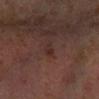Notes:
– follow-up · imaged on a skin check; not biopsied
– subject · male, aged 63–67
– image · total-body-photography crop, ~15 mm field of view
– diameter · ~2.5 mm (longest diameter)
– body site · the left forearm
– automated lesion analysis · a classifier nevus-likeness of about 15/100 and a detector confidence of about 95 out of 100 that the crop contains a lesion